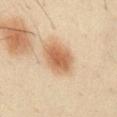– notes — no biopsy performed (imaged during a skin exam)
– site — the chest
– subject — male, aged around 50
– TBP lesion metrics — a footprint of about 13 mm² and a symmetry-axis asymmetry near 0.1
– lesion size — ~5 mm (longest diameter)
– image — ~15 mm tile from a whole-body skin photo
– lighting — cross-polarized illumination